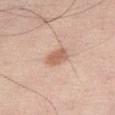Clinical summary: Captured under white-light illumination. The recorded lesion diameter is about 3 mm. A region of skin cropped from a whole-body photographic capture, roughly 15 mm wide. From the leg. The lesion-visualizer software estimated an area of roughly 5 mm², an outline eccentricity of about 0.75 (0 = round, 1 = elongated), and a symmetry-axis asymmetry near 0.2. The software also gave a border-irregularity rating of about 2/10, a color-variation rating of about 2/10, and a peripheral color-asymmetry measure near 0.5. The patient is a male aged around 70.